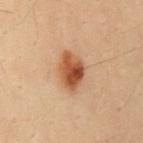The lesion was photographed on a routine skin check and not biopsied; there is no pathology result. A 15 mm crop from a total-body photograph taken for skin-cancer surveillance. This is a cross-polarized tile. Approximately 4 mm at its widest. A male patient, aged around 30. Located on the mid back.